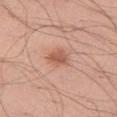Recorded during total-body skin imaging; not selected for excision or biopsy. Captured under white-light illumination. A close-up tile cropped from a whole-body skin photograph, about 15 mm across. A male subject, aged around 40. Located on the left thigh.